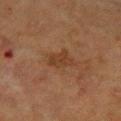Q: Is there a histopathology result?
A: catalogued during a skin exam; not biopsied
Q: Automated lesion metrics?
A: lesion-presence confidence of about 100/100
Q: What are the patient's age and sex?
A: female, in their 80s
Q: Lesion location?
A: the right forearm
Q: How was this image acquired?
A: total-body-photography crop, ~15 mm field of view
Q: How was the tile lit?
A: cross-polarized
Q: How large is the lesion?
A: ≈3 mm